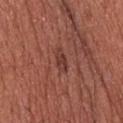Context: The lesion is on the head or neck. A male patient, in their mid-50s. A 15 mm close-up extracted from a 3D total-body photography capture. The total-body-photography lesion software estimated border irregularity of about 3 on a 0–10 scale, a color-variation rating of about 1.5/10, and peripheral color asymmetry of about 0.5. The analysis additionally found a nevus-likeness score of about 15/100 and a lesion-detection confidence of about 100/100. Imaged with white-light lighting. About 3 mm across.